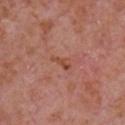Longest diameter approximately 2.5 mm. A region of skin cropped from a whole-body photographic capture, roughly 15 mm wide. A male patient, aged around 65. Captured under white-light illumination. An algorithmic analysis of the crop reported a lesion area of about 2.5 mm², an outline eccentricity of about 0.9 (0 = round, 1 = elongated), and a shape-asymmetry score of about 0.45 (0 = symmetric). The software also gave about 7 CIELAB-L* units darker than the surrounding skin and a normalized border contrast of about 6.5. It also reported a within-lesion color-variation index near 1.5/10 and radial color variation of about 0.5. The lesion is on the front of the torso.